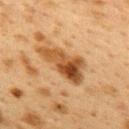Clinical impression: Imaged during a routine full-body skin examination; the lesion was not biopsied and no histopathology is available. Context: The subject is a female aged approximately 40. Located on the upper back. A lesion tile, about 15 mm wide, cut from a 3D total-body photograph.